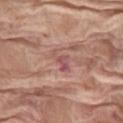Recorded during total-body skin imaging; not selected for excision or biopsy.
The lesion-visualizer software estimated an area of roughly 3.5 mm², an outline eccentricity of about 0.9 (0 = round, 1 = elongated), and a shape-asymmetry score of about 0.5 (0 = symmetric). The software also gave a mean CIELAB color near L≈52 a*≈26 b*≈21 and roughly 9 lightness units darker than nearby skin. The analysis additionally found an automated nevus-likeness rating near 0 out of 100.
A lesion tile, about 15 mm wide, cut from a 3D total-body photograph.
About 3.5 mm across.
From the leg.
A female patient, in their mid-70s.
Captured under white-light illumination.